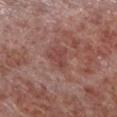The lesion was photographed on a routine skin check and not biopsied; there is no pathology result. On the left lower leg. The patient is a male about 55 years old. A lesion tile, about 15 mm wide, cut from a 3D total-body photograph.